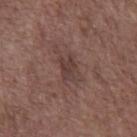{
  "biopsy_status": "not biopsied; imaged during a skin examination",
  "site": "abdomen",
  "lesion_size": {
    "long_diameter_mm_approx": 3.0
  },
  "patient": {
    "sex": "male",
    "age_approx": 70
  },
  "lighting": "white-light",
  "image": {
    "source": "total-body photography crop",
    "field_of_view_mm": 15
  }
}